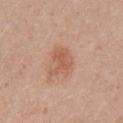Located on the arm. Longest diameter approximately 3.5 mm. A 15 mm close-up tile from a total-body photography series done for melanoma screening. The tile uses white-light illumination. The subject is a male aged approximately 25.